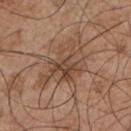Q: Was this lesion biopsied?
A: total-body-photography surveillance lesion; no biopsy
Q: Who is the patient?
A: male, about 55 years old
Q: What kind of image is this?
A: total-body-photography crop, ~15 mm field of view
Q: What is the anatomic site?
A: the front of the torso